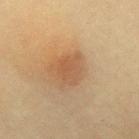Image and clinical context:
A female subject about 60 years old. Automated image analysis of the tile measured border irregularity of about 3 on a 0–10 scale, a color-variation rating of about 3/10, and a peripheral color-asymmetry measure near 1. The software also gave a nevus-likeness score of about 65/100 and a lesion-detection confidence of about 100/100. A 15 mm crop from a total-body photograph taken for skin-cancer surveillance. The lesion is located on the mid back. The recorded lesion diameter is about 3.5 mm. This is a cross-polarized tile.